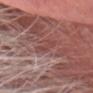workup=total-body-photography surveillance lesion; no biopsy